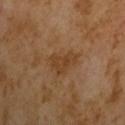Case summary:
• follow-up — imaged on a skin check; not biopsied
• illumination — cross-polarized illumination
• diameter — about 4 mm
• acquisition — ~15 mm tile from a whole-body skin photo
• patient — male, aged approximately 65